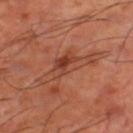The lesion was tiled from a total-body skin photograph and was not biopsied. A close-up tile cropped from a whole-body skin photograph, about 15 mm across. This is a cross-polarized tile. The lesion's longest dimension is about 6.5 mm. From the left thigh. The subject is aged 63–67.